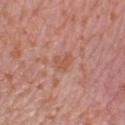<tbp_lesion>
<biopsy_status>not biopsied; imaged during a skin examination</biopsy_status>
</tbp_lesion>